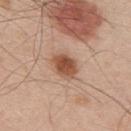biopsy status: imaged on a skin check; not biopsied | lighting: white-light illumination | subject: male, aged 48–52 | anatomic site: the back | lesion diameter: about 3.5 mm | imaging modality: total-body-photography crop, ~15 mm field of view.